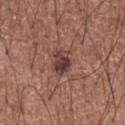Assessment:
The lesion was tiled from a total-body skin photograph and was not biopsied.
Context:
The lesion is located on the chest. A male patient aged 73–77. A roughly 15 mm field-of-view crop from a total-body skin photograph. The tile uses white-light illumination. The total-body-photography lesion software estimated an area of roughly 5 mm², a shape eccentricity near 0.75, and a symmetry-axis asymmetry near 0.25.